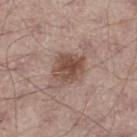biopsy status: no biopsy performed (imaged during a skin exam) | image: 15 mm crop, total-body photography | subject: male, aged approximately 55 | lesion diameter: ~4 mm (longest diameter) | body site: the left thigh | illumination: white-light illumination.